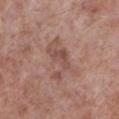notes = total-body-photography surveillance lesion; no biopsy
acquisition = 15 mm crop, total-body photography
body site = the left lower leg
patient = male, aged 53 to 57
size = ~5 mm (longest diameter)